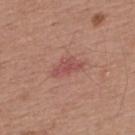Recorded during total-body skin imaging; not selected for excision or biopsy. A male patient roughly 55 years of age. Captured under white-light illumination. The lesion is on the upper back. A close-up tile cropped from a whole-body skin photograph, about 15 mm across.